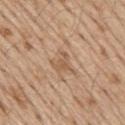Context: On the mid back. Approximately 2.5 mm at its widest. Captured under white-light illumination. A male subject, in their 70s. A roughly 15 mm field-of-view crop from a total-body skin photograph.